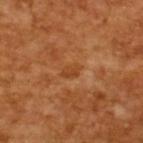Findings:
– notes · total-body-photography surveillance lesion; no biopsy
– automated metrics · a lesion-to-skin contrast of about 5.5 (normalized; higher = more distinct); a detector confidence of about 100 out of 100 that the crop contains a lesion
– image · ~15 mm tile from a whole-body skin photo
– patient · male, approximately 65 years of age
– lesion diameter · ≈2.5 mm
– illumination · cross-polarized